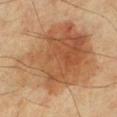  lighting: cross-polarized
  automated_metrics:
    cielab_L: 52
    cielab_a: 20
    cielab_b: 34
    vs_skin_darker_L: 11.0
    vs_skin_contrast_norm: 8.0
    border_irregularity_0_10: 4.5
    color_variation_0_10: 6.5
    peripheral_color_asymmetry: 2.0
  image:
    source: total-body photography crop
    field_of_view_mm: 15
  lesion_size:
    long_diameter_mm_approx: 11.0
  patient:
    sex: male
    age_approx: 70
  site: leg